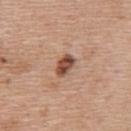- follow-up · imaged on a skin check; not biopsied
- diameter · about 3 mm
- patient · male, in their 60s
- anatomic site · the upper back
- image · ~15 mm crop, total-body skin-cancer survey
- illumination · white-light illumination